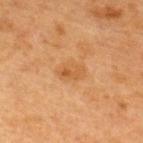The lesion-visualizer software estimated an area of roughly 5.5 mm². The analysis additionally found a classifier nevus-likeness of about 5/100 and a lesion-detection confidence of about 100/100.
The lesion is on the upper back.
The subject is a female approximately 40 years of age.
The recorded lesion diameter is about 3 mm.
A roughly 15 mm field-of-view crop from a total-body skin photograph.